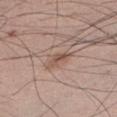This lesion was catalogued during total-body skin photography and was not selected for biopsy.
A male subject aged approximately 30.
Approximately 2.5 mm at its widest.
A region of skin cropped from a whole-body photographic capture, roughly 15 mm wide.
From the left forearm.
Automated tile analysis of the lesion measured an area of roughly 2.5 mm², an eccentricity of roughly 0.85, and a shape-asymmetry score of about 0.45 (0 = symmetric). The software also gave a mean CIELAB color near L≈52 a*≈18 b*≈25, about 9 CIELAB-L* units darker than the surrounding skin, and a normalized border contrast of about 7.